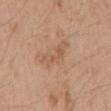workup: no biopsy performed (imaged during a skin exam); automated metrics: a border-irregularity rating of about 6.5/10 and internal color variation of about 3 on a 0–10 scale; image source: 15 mm crop, total-body photography; lesion diameter: ≈5 mm; patient: male, approximately 45 years of age; lighting: white-light; site: the mid back.